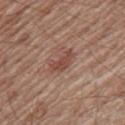Impression:
No biopsy was performed on this lesion — it was imaged during a full skin examination and was not determined to be concerning.
Image and clinical context:
Automated image analysis of the tile measured a normalized lesion–skin contrast near 6.5. The software also gave a border-irregularity index near 3.5/10, a color-variation rating of about 2/10, and a peripheral color-asymmetry measure near 0.5. The analysis additionally found a nevus-likeness score of about 30/100 and a lesion-detection confidence of about 100/100. On the right thigh. Measured at roughly 3.5 mm in maximum diameter. The patient is a male aged 53–57. A region of skin cropped from a whole-body photographic capture, roughly 15 mm wide.